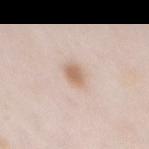- location: the chest
- size: ~2.5 mm (longest diameter)
- image: ~15 mm tile from a whole-body skin photo
- subject: female, roughly 65 years of age
- illumination: white-light